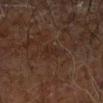– workup: no biopsy performed (imaged during a skin exam)
– site: the left forearm
– imaging modality: ~15 mm tile from a whole-body skin photo
– size: ~2.5 mm (longest diameter)
– illumination: cross-polarized
– subject: male, aged approximately 70